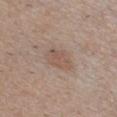Assessment: Part of a total-body skin-imaging series; this lesion was reviewed on a skin check and was not flagged for biopsy. Background: This is a white-light tile. The subject is a male approximately 40 years of age. A roughly 15 mm field-of-view crop from a total-body skin photograph. Automated tile analysis of the lesion measured an area of roughly 7.5 mm², an eccentricity of roughly 0.7, and a symmetry-axis asymmetry near 0.2. And it measured a mean CIELAB color near L≈54 a*≈16 b*≈25, about 7 CIELAB-L* units darker than the surrounding skin, and a normalized lesion–skin contrast near 5. The lesion is located on the chest. About 3.5 mm across.